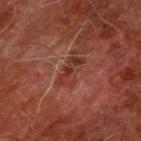The lesion was tiled from a total-body skin photograph and was not biopsied. The total-body-photography lesion software estimated an area of roughly 4.5 mm², an eccentricity of roughly 0.9, and two-axis asymmetry of about 0.4. And it measured a border-irregularity index near 6/10, internal color variation of about 0.5 on a 0–10 scale, and peripheral color asymmetry of about 0. A lesion tile, about 15 mm wide, cut from a 3D total-body photograph. About 4 mm across. On the left forearm. The patient is a male about 80 years old.